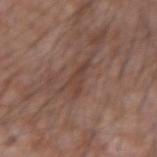Impression: The lesion was photographed on a routine skin check and not biopsied; there is no pathology result. Context: A region of skin cropped from a whole-body photographic capture, roughly 15 mm wide. A male subject approximately 55 years of age. The tile uses white-light illumination. Measured at roughly 4 mm in maximum diameter. From the left forearm.